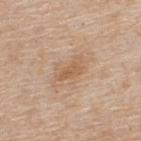Assessment:
Recorded during total-body skin imaging; not selected for excision or biopsy.
Acquisition and patient details:
The subject is a male aged 53–57. A roughly 15 mm field-of-view crop from a total-body skin photograph. On the upper back. Measured at roughly 3.5 mm in maximum diameter.